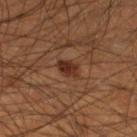The lesion was tiled from a total-body skin photograph and was not biopsied. A region of skin cropped from a whole-body photographic capture, roughly 15 mm wide. Located on the leg. A male patient, roughly 45 years of age. Captured under cross-polarized illumination. The lesion's longest dimension is about 2.5 mm.